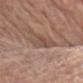Assessment:
Imaged during a routine full-body skin examination; the lesion was not biopsied and no histopathology is available.
Clinical summary:
A region of skin cropped from a whole-body photographic capture, roughly 15 mm wide. The patient is a male aged approximately 65. Approximately 4.5 mm at its widest. Captured under white-light illumination. An algorithmic analysis of the crop reported an average lesion color of about L≈50 a*≈18 b*≈25 (CIELAB) and a lesion-to-skin contrast of about 6 (normalized; higher = more distinct). The software also gave a border-irregularity rating of about 3/10. The analysis additionally found lesion-presence confidence of about 75/100. On the right upper arm.